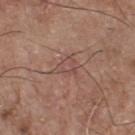Q: Was this lesion biopsied?
A: no biopsy performed (imaged during a skin exam)
Q: Lesion location?
A: the chest
Q: Lesion size?
A: ≈3 mm
Q: What lighting was used for the tile?
A: white-light illumination
Q: What are the patient's age and sex?
A: male, in their mid-70s
Q: How was this image acquired?
A: 15 mm crop, total-body photography
Q: Automated lesion metrics?
A: a lesion color around L≈49 a*≈20 b*≈23 in CIELAB, a lesion–skin lightness drop of about 6, and a lesion-to-skin contrast of about 4.5 (normalized; higher = more distinct); border irregularity of about 4 on a 0–10 scale, internal color variation of about 2.5 on a 0–10 scale, and a peripheral color-asymmetry measure near 1; an automated nevus-likeness rating near 0 out of 100 and a lesion-detection confidence of about 75/100